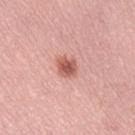- biopsy status · catalogued during a skin exam; not biopsied
- lighting · white-light illumination
- subject · female, aged around 40
- body site · the leg
- acquisition · ~15 mm tile from a whole-body skin photo
- TBP lesion metrics · a border-irregularity index near 2/10, a color-variation rating of about 3/10, and a peripheral color-asymmetry measure near 1